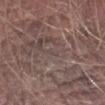Clinical impression:
Imaged during a routine full-body skin examination; the lesion was not biopsied and no histopathology is available.
Acquisition and patient details:
An algorithmic analysis of the crop reported a border-irregularity rating of about 4.5/10 and a color-variation rating of about 0/10. It also reported a nevus-likeness score of about 0/100 and a lesion-detection confidence of about 0/100. From the right forearm. A close-up tile cropped from a whole-body skin photograph, about 15 mm across. Longest diameter approximately 1.5 mm. A male subject, roughly 75 years of age. Captured under white-light illumination.